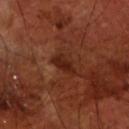Automated image analysis of the tile measured a lesion area of about 5 mm², an eccentricity of roughly 0.8, and a shape-asymmetry score of about 0.3 (0 = symmetric). And it measured a mean CIELAB color near L≈24 a*≈24 b*≈27, a lesion–skin lightness drop of about 8, and a normalized lesion–skin contrast near 8.5. And it measured a border-irregularity index near 3/10, internal color variation of about 2 on a 0–10 scale, and peripheral color asymmetry of about 0.5. And it measured a classifier nevus-likeness of about 5/100 and a detector confidence of about 100 out of 100 that the crop contains a lesion. The lesion is located on the front of the torso. A 15 mm close-up tile from a total-body photography series done for melanoma screening. A male patient roughly 70 years of age. The tile uses cross-polarized illumination. Approximately 3 mm at its widest.